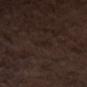Findings:
* biopsy status: imaged on a skin check; not biopsied
* tile lighting: white-light illumination
* imaging modality: ~15 mm crop, total-body skin-cancer survey
* patient: male, aged around 70
* anatomic site: the chest